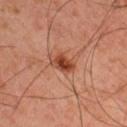notes — total-body-photography surveillance lesion; no biopsy
location — the arm
automated metrics — an area of roughly 5.5 mm², an eccentricity of roughly 0.65, and a shape-asymmetry score of about 0.25 (0 = symmetric); an average lesion color of about L≈43 a*≈27 b*≈32 (CIELAB), roughly 12 lightness units darker than nearby skin, and a normalized border contrast of about 9.5; border irregularity of about 2.5 on a 0–10 scale, a color-variation rating of about 5.5/10, and peripheral color asymmetry of about 2
illumination — cross-polarized illumination
image — total-body-photography crop, ~15 mm field of view
size — ≈3 mm
patient — male, roughly 45 years of age